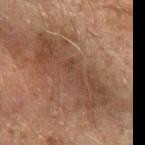Notes:
• notes: imaged on a skin check; not biopsied
• location: the left forearm
• size: ~10.5 mm (longest diameter)
• illumination: cross-polarized
• subject: male, aged approximately 65
• automated lesion analysis: an area of roughly 31 mm² and a shape-asymmetry score of about 0.3 (0 = symmetric); a border-irregularity rating of about 6/10, a within-lesion color-variation index near 3.5/10, and a peripheral color-asymmetry measure near 1
• image source: ~15 mm tile from a whole-body skin photo